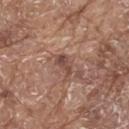| feature | finding |
|---|---|
| biopsy status | total-body-photography surveillance lesion; no biopsy |
| size | ≈3 mm |
| image | ~15 mm crop, total-body skin-cancer survey |
| patient | male, aged 78–82 |
| lighting | white-light illumination |
| location | the back |